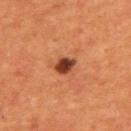Q: Was this lesion biopsied?
A: no biopsy performed (imaged during a skin exam)
Q: What is the imaging modality?
A: ~15 mm crop, total-body skin-cancer survey
Q: Who is the patient?
A: male, aged 53 to 57
Q: What is the lesion's diameter?
A: ≈2.5 mm
Q: Lesion location?
A: the upper back